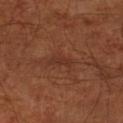Q: Is there a histopathology result?
A: total-body-photography surveillance lesion; no biopsy
Q: Where on the body is the lesion?
A: the left lower leg
Q: How was the tile lit?
A: cross-polarized
Q: Lesion size?
A: ≈2.5 mm
Q: What did automated image analysis measure?
A: an area of roughly 3 mm² and a symmetry-axis asymmetry near 0.4; roughly 5 lightness units darker than nearby skin and a normalized border contrast of about 5.5; a border-irregularity index near 4/10, internal color variation of about 0 on a 0–10 scale, and radial color variation of about 0
Q: How was this image acquired?
A: ~15 mm tile from a whole-body skin photo
Q: What are the patient's age and sex?
A: aged around 65